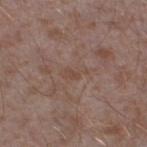- biopsy status — total-body-photography surveillance lesion; no biopsy
- subject — male, roughly 45 years of age
- lesion size — ≈3 mm
- tile lighting — white-light
- acquisition — ~15 mm tile from a whole-body skin photo
- body site — the left lower leg